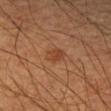Clinical impression:
Part of a total-body skin-imaging series; this lesion was reviewed on a skin check and was not flagged for biopsy.
Context:
A male subject, aged around 55. From the left forearm. The recorded lesion diameter is about 2.5 mm. This is a cross-polarized tile. A region of skin cropped from a whole-body photographic capture, roughly 15 mm wide.